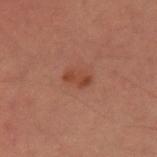{"biopsy_status": "not biopsied; imaged during a skin examination"}